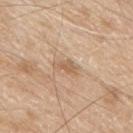Impression:
Captured during whole-body skin photography for melanoma surveillance; the lesion was not biopsied.
Background:
The subject is a male approximately 80 years of age. Located on the upper back. A 15 mm close-up extracted from a 3D total-body photography capture.